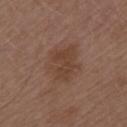Notes:
* notes: catalogued during a skin exam; not biopsied
* lighting: white-light illumination
* subject: male, aged 48–52
* acquisition: ~15 mm crop, total-body skin-cancer survey
* size: ~4.5 mm (longest diameter)
* body site: the left upper arm